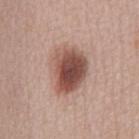workup=total-body-photography surveillance lesion; no biopsy | subject=female, roughly 45 years of age | acquisition=total-body-photography crop, ~15 mm field of view | body site=the abdomen.